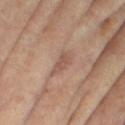Recorded during total-body skin imaging; not selected for excision or biopsy. The recorded lesion diameter is about 3 mm. The total-body-photography lesion software estimated a lesion color around L≈53 a*≈20 b*≈27 in CIELAB and about 8 CIELAB-L* units darker than the surrounding skin. The analysis additionally found a color-variation rating of about 1.5/10 and peripheral color asymmetry of about 0.5. And it measured an automated nevus-likeness rating near 0 out of 100 and a detector confidence of about 100 out of 100 that the crop contains a lesion. A female patient roughly 75 years of age. A lesion tile, about 15 mm wide, cut from a 3D total-body photograph. Located on the leg.